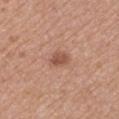{
  "lesion_size": {
    "long_diameter_mm_approx": 2.5
  },
  "automated_metrics": {
    "cielab_L": 52,
    "cielab_a": 23,
    "cielab_b": 29,
    "vs_skin_darker_L": 10.0,
    "vs_skin_contrast_norm": 7.0,
    "border_irregularity_0_10": 2.0,
    "color_variation_0_10": 2.0
  },
  "lighting": "white-light",
  "patient": {
    "sex": "male",
    "age_approx": 55
  },
  "site": "right upper arm",
  "image": {
    "source": "total-body photography crop",
    "field_of_view_mm": 15
  }
}